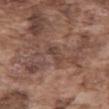Assessment:
The lesion was tiled from a total-body skin photograph and was not biopsied.
Acquisition and patient details:
A male subject in their mid- to late 70s. The tile uses white-light illumination. Cropped from a total-body skin-imaging series; the visible field is about 15 mm. On the abdomen.